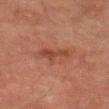Q: What is the anatomic site?
A: the left thigh
Q: What is the imaging modality?
A: 15 mm crop, total-body photography
Q: What is the lesion's diameter?
A: about 5 mm
Q: Patient demographics?
A: male, aged 73 to 77
Q: Illumination type?
A: cross-polarized